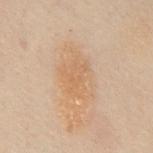  image:
    source: total-body photography crop
    field_of_view_mm: 15
  site: abdomen
  lesion_size:
    long_diameter_mm_approx: 5.5
  automated_metrics:
    area_mm2_approx: 13.0
    eccentricity: 0.75
    shape_asymmetry: 0.2
    cielab_L: 52
    cielab_a: 15
    cielab_b: 30
    border_irregularity_0_10: 2.5
    peripheral_color_asymmetry: 1.0
    nevus_likeness_0_100: 20
    lesion_detection_confidence_0_100: 100
  lighting: cross-polarized
  patient:
    sex: male
    age_approx: 50